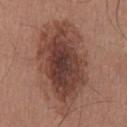Q: Is there a histopathology result?
A: total-body-photography surveillance lesion; no biopsy
Q: How large is the lesion?
A: ~11.5 mm (longest diameter)
Q: What is the imaging modality?
A: 15 mm crop, total-body photography
Q: What is the anatomic site?
A: the back
Q: What are the patient's age and sex?
A: male, aged approximately 40